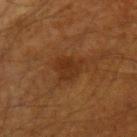This lesion was catalogued during total-body skin photography and was not selected for biopsy.
Cropped from a whole-body photographic skin survey; the tile spans about 15 mm.
From the left upper arm.
A male subject, aged 58 to 62.